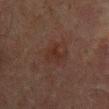Context:
The recorded lesion diameter is about 3 mm. A 15 mm crop from a total-body photograph taken for skin-cancer surveillance. Captured under cross-polarized illumination. A male patient, aged approximately 65. The lesion is located on the mid back. Automated tile analysis of the lesion measured a footprint of about 4.5 mm², a shape eccentricity near 0.7, and two-axis asymmetry of about 0.55. The analysis additionally found about 4 CIELAB-L* units darker than the surrounding skin. The software also gave border irregularity of about 5.5 on a 0–10 scale, a within-lesion color-variation index near 1.5/10, and radial color variation of about 0.5.